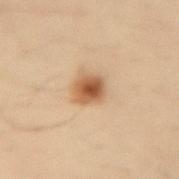follow-up = total-body-photography surveillance lesion; no biopsy
imaging modality = total-body-photography crop, ~15 mm field of view
tile lighting = cross-polarized
subject = female, in their mid- to late 30s
body site = the right forearm
diameter = about 3 mm